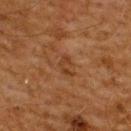notes: catalogued during a skin exam; not biopsied | image source: ~15 mm crop, total-body skin-cancer survey | subject: male, about 60 years old | illumination: cross-polarized illumination | location: the back.